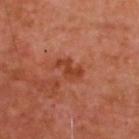Notes:
– workup · imaged on a skin check; not biopsied
– diameter · ≈3.5 mm
– image source · ~15 mm tile from a whole-body skin photo
– image-analysis metrics · a lesion color around L≈43 a*≈30 b*≈35 in CIELAB and a lesion–skin lightness drop of about 9; border irregularity of about 4 on a 0–10 scale, a color-variation rating of about 2/10, and peripheral color asymmetry of about 0.5; a detector confidence of about 100 out of 100 that the crop contains a lesion
– subject · male, aged 48–52
– location · the upper back